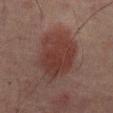No biopsy was performed on this lesion — it was imaged during a full skin examination and was not determined to be concerning.
Approximately 7 mm at its widest.
A male patient, about 65 years old.
Located on the mid back.
Imaged with cross-polarized lighting.
A close-up tile cropped from a whole-body skin photograph, about 15 mm across.
The total-body-photography lesion software estimated a lesion area of about 27 mm², a shape eccentricity near 0.65, and a shape-asymmetry score of about 0.15 (0 = symmetric). The software also gave border irregularity of about 2 on a 0–10 scale and a color-variation rating of about 3.5/10. And it measured an automated nevus-likeness rating near 95 out of 100 and a lesion-detection confidence of about 100/100.